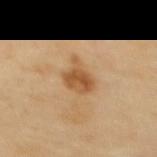Assessment: Imaged during a routine full-body skin examination; the lesion was not biopsied and no histopathology is available. Clinical summary: A female subject, in their 60s. Located on the upper back. About 3.5 mm across. A close-up tile cropped from a whole-body skin photograph, about 15 mm across.